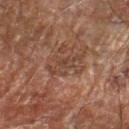Q: Is there a histopathology result?
A: catalogued during a skin exam; not biopsied
Q: Who is the patient?
A: male, in their mid-60s
Q: What lighting was used for the tile?
A: cross-polarized illumination
Q: Lesion location?
A: the left forearm
Q: What is the lesion's diameter?
A: ~4 mm (longest diameter)
Q: What kind of image is this?
A: total-body-photography crop, ~15 mm field of view